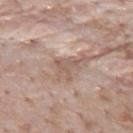follow-up: catalogued during a skin exam; not biopsied | lesion size: about 3 mm | lighting: white-light illumination | automated lesion analysis: a lesion area of about 3.5 mm², an outline eccentricity of about 0.35 (0 = round, 1 = elongated), and a shape-asymmetry score of about 0.65 (0 = symmetric); a lesion color around L≈56 a*≈17 b*≈26 in CIELAB, about 8 CIELAB-L* units darker than the surrounding skin, and a lesion-to-skin contrast of about 5.5 (normalized; higher = more distinct); a border-irregularity rating of about 8/10, internal color variation of about 0 on a 0–10 scale, and peripheral color asymmetry of about 0; an automated nevus-likeness rating near 0 out of 100 and a lesion-detection confidence of about 95/100 | imaging modality: ~15 mm crop, total-body skin-cancer survey | site: the mid back | patient: male, about 55 years old.